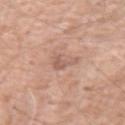Recorded during total-body skin imaging; not selected for excision or biopsy. Captured under white-light illumination. An algorithmic analysis of the crop reported an area of roughly 4 mm², an outline eccentricity of about 0.8 (0 = round, 1 = elongated), and a shape-asymmetry score of about 0.6 (0 = symmetric). And it measured border irregularity of about 6 on a 0–10 scale and a within-lesion color-variation index near 1/10. About 3.5 mm across. On the arm. A male subject approximately 50 years of age. Cropped from a total-body skin-imaging series; the visible field is about 15 mm.